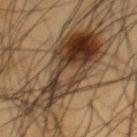Findings:
* workup: total-body-photography surveillance lesion; no biopsy
* imaging modality: ~15 mm crop, total-body skin-cancer survey
* lesion size: about 10.5 mm
* subject: male, about 60 years old
* tile lighting: cross-polarized
* site: the front of the torso